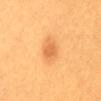Clinical impression: Recorded during total-body skin imaging; not selected for excision or biopsy. Context: A female subject, aged around 30. Cropped from a total-body skin-imaging series; the visible field is about 15 mm. Longest diameter approximately 3 mm. An algorithmic analysis of the crop reported a footprint of about 4.5 mm², a shape eccentricity near 0.75, and a symmetry-axis asymmetry near 0.25. It also reported a lesion–skin lightness drop of about 9 and a normalized lesion–skin contrast near 6. The software also gave a border-irregularity index near 2.5/10, a color-variation rating of about 1/10, and a peripheral color-asymmetry measure near 0.5. It also reported an automated nevus-likeness rating near 100 out of 100 and a detector confidence of about 100 out of 100 that the crop contains a lesion. From the abdomen. Captured under cross-polarized illumination.